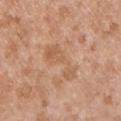lighting: white-light
anatomic site: the front of the torso
patient: male, roughly 35 years of age
acquisition: total-body-photography crop, ~15 mm field of view